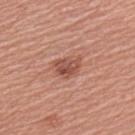The lesion was photographed on a routine skin check and not biopsied; there is no pathology result. A 15 mm crop from a total-body photograph taken for skin-cancer surveillance. A female subject aged 48 to 52. The recorded lesion diameter is about 3 mm. Located on the left upper arm. Automated tile analysis of the lesion measured an area of roughly 5.5 mm². The software also gave a mean CIELAB color near L≈51 a*≈25 b*≈28 and roughly 11 lightness units darker than nearby skin. The software also gave a nevus-likeness score of about 55/100. Imaged with white-light lighting.